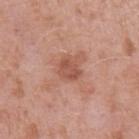Clinical impression:
This lesion was catalogued during total-body skin photography and was not selected for biopsy.
Image and clinical context:
A male patient aged 38–42. Imaged with white-light lighting. A close-up tile cropped from a whole-body skin photograph, about 15 mm across. The lesion is on the left upper arm.